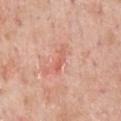notes: catalogued during a skin exam; not biopsied
body site: the chest
diameter: ≈3 mm
subject: male, roughly 60 years of age
image source: 15 mm crop, total-body photography
automated metrics: a footprint of about 3 mm², an outline eccentricity of about 0.95 (0 = round, 1 = elongated), and a shape-asymmetry score of about 0.25 (0 = symmetric); a within-lesion color-variation index near 0/10 and radial color variation of about 0; a detector confidence of about 100 out of 100 that the crop contains a lesion